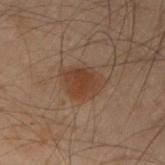TBP lesion metrics: a border-irregularity index near 2/10, a color-variation rating of about 2.5/10, and a peripheral color-asymmetry measure near 0.5; an automated nevus-likeness rating near 75 out of 100
patient: male, aged 48–52
site: the arm
tile lighting: cross-polarized illumination
lesion size: ~4 mm (longest diameter)
acquisition: 15 mm crop, total-body photography